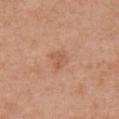Impression: Imaged during a routine full-body skin examination; the lesion was not biopsied and no histopathology is available. Clinical summary: The lesion is on the chest. A lesion tile, about 15 mm wide, cut from a 3D total-body photograph. This is a white-light tile. A female subject aged 58–62.